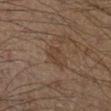Q: Was this lesion biopsied?
A: catalogued during a skin exam; not biopsied
Q: How was this image acquired?
A: ~15 mm crop, total-body skin-cancer survey
Q: What lighting was used for the tile?
A: cross-polarized
Q: Patient demographics?
A: male, in their mid- to late 60s
Q: Lesion size?
A: about 2.5 mm
Q: Lesion location?
A: the right lower leg